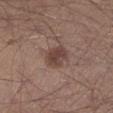biopsy status = imaged on a skin check; not biopsied | patient = male, aged approximately 30 | location = the left lower leg | acquisition = ~15 mm crop, total-body skin-cancer survey.